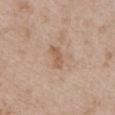| key | value |
|---|---|
| biopsy status | total-body-photography surveillance lesion; no biopsy |
| body site | the left upper arm |
| subject | male, aged 48 to 52 |
| TBP lesion metrics | a footprint of about 3.5 mm², an eccentricity of roughly 0.85, and two-axis asymmetry of about 0.3; a lesion color around L≈57 a*≈18 b*≈31 in CIELAB and a normalized lesion–skin contrast near 6 |
| image | total-body-photography crop, ~15 mm field of view |